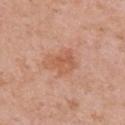Image and clinical context: The lesion is located on the chest. This is a white-light tile. The patient is a female aged approximately 50. A 15 mm crop from a total-body photograph taken for skin-cancer surveillance.